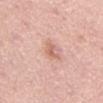Impression:
Captured during whole-body skin photography for melanoma surveillance; the lesion was not biopsied.
Acquisition and patient details:
From the abdomen. The subject is a male about 55 years old. Measured at roughly 2.5 mm in maximum diameter. Automated tile analysis of the lesion measured a footprint of about 3.5 mm², an outline eccentricity of about 0.75 (0 = round, 1 = elongated), and a shape-asymmetry score of about 0.3 (0 = symmetric). The analysis additionally found a lesion color around L≈64 a*≈23 b*≈29 in CIELAB. It also reported a border-irregularity rating of about 3.5/10, a color-variation rating of about 2.5/10, and radial color variation of about 1. It also reported a lesion-detection confidence of about 100/100. A 15 mm crop from a total-body photograph taken for skin-cancer surveillance. Imaged with white-light lighting.